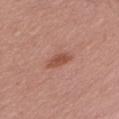Imaged during a routine full-body skin examination; the lesion was not biopsied and no histopathology is available. A female subject aged 48–52. A 15 mm close-up extracted from a 3D total-body photography capture. The tile uses white-light illumination. The lesion is located on the arm. About 3 mm across.